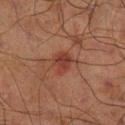<tbp_lesion>
<biopsy_status>not biopsied; imaged during a skin examination</biopsy_status>
<lesion_size>
  <long_diameter_mm_approx>3.0</long_diameter_mm_approx>
</lesion_size>
<automated_metrics>
  <area_mm2_approx>6.5</area_mm2_approx>
  <eccentricity>0.55</eccentricity>
  <shape_asymmetry>0.2</shape_asymmetry>
  <cielab_L>33</cielab_L>
  <cielab_a>22</cielab_a>
  <cielab_b>24</cielab_b>
  <vs_skin_darker_L>7.0</vs_skin_darker_L>
  <vs_skin_contrast_norm>6.5</vs_skin_contrast_norm>
  <nevus_likeness_0_100>30</nevus_likeness_0_100>
  <lesion_detection_confidence_0_100>100</lesion_detection_confidence_0_100>
</automated_metrics>
<patient>
  <sex>male</sex>
  <age_approx>70</age_approx>
</patient>
<site>right lower leg</site>
<lighting>cross-polarized</lighting>
<image>
  <source>total-body photography crop</source>
  <field_of_view_mm>15</field_of_view_mm>
</image>
</tbp_lesion>